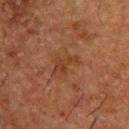Assessment:
No biopsy was performed on this lesion — it was imaged during a full skin examination and was not determined to be concerning.
Image and clinical context:
Cropped from a whole-body photographic skin survey; the tile spans about 15 mm. A male subject, about 50 years old. The lesion is on the upper back. Longest diameter approximately 3.5 mm. The tile uses cross-polarized illumination. The total-body-photography lesion software estimated a lesion area of about 4 mm², an eccentricity of roughly 0.85, and two-axis asymmetry of about 0.55. It also reported border irregularity of about 6.5 on a 0–10 scale, a color-variation rating of about 2/10, and a peripheral color-asymmetry measure near 1. The analysis additionally found an automated nevus-likeness rating near 0 out of 100.